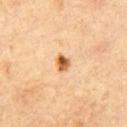| feature | finding |
|---|---|
| biopsy status | catalogued during a skin exam; not biopsied |
| acquisition | total-body-photography crop, ~15 mm field of view |
| size | ≈2 mm |
| tile lighting | cross-polarized illumination |
| image-analysis metrics | a lesion area of about 3 mm², an eccentricity of roughly 0.6, and a shape-asymmetry score of about 0.25 (0 = symmetric); a mean CIELAB color near L≈60 a*≈24 b*≈43, about 17 CIELAB-L* units darker than the surrounding skin, and a normalized lesion–skin contrast near 11; a border-irregularity rating of about 2/10, a within-lesion color-variation index near 8/10, and peripheral color asymmetry of about 2.5 |
| subject | male, aged around 60 |
| site | the chest |